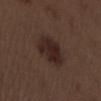Background:
This is a white-light tile. The lesion-visualizer software estimated a footprint of about 12 mm², an outline eccentricity of about 0.6 (0 = round, 1 = elongated), and two-axis asymmetry of about 0.15. It also reported border irregularity of about 2 on a 0–10 scale, a color-variation rating of about 3.5/10, and radial color variation of about 1. The analysis additionally found a nevus-likeness score of about 80/100 and a detector confidence of about 100 out of 100 that the crop contains a lesion. The lesion is on the left upper arm. The subject is a male about 70 years old. A close-up tile cropped from a whole-body skin photograph, about 15 mm across.